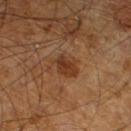Captured during whole-body skin photography for melanoma surveillance; the lesion was not biopsied. The tile uses cross-polarized illumination. A male patient aged around 65. A 15 mm close-up extracted from a 3D total-body photography capture. Located on the leg. Automated tile analysis of the lesion measured a footprint of about 6.5 mm² and an eccentricity of roughly 0.7. The analysis additionally found a lesion color around L≈36 a*≈21 b*≈32 in CIELAB, roughly 8 lightness units darker than nearby skin, and a lesion-to-skin contrast of about 7.5 (normalized; higher = more distinct). It also reported a border-irregularity index near 2.5/10, a within-lesion color-variation index near 3/10, and a peripheral color-asymmetry measure near 1. The analysis additionally found a classifier nevus-likeness of about 75/100 and a detector confidence of about 100 out of 100 that the crop contains a lesion.